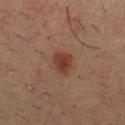Image and clinical context:
The lesion's longest dimension is about 2.5 mm. On the chest. A lesion tile, about 15 mm wide, cut from a 3D total-body photograph. The total-body-photography lesion software estimated a border-irregularity rating of about 2/10 and internal color variation of about 2.5 on a 0–10 scale. A male subject, in their mid-30s.